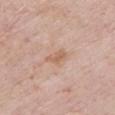Notes:
- workup · catalogued during a skin exam; not biopsied
- body site · the chest
- patient · female, aged approximately 70
- image · total-body-photography crop, ~15 mm field of view
- automated metrics · a footprint of about 3.5 mm², an outline eccentricity of about 0.85 (0 = round, 1 = elongated), and a symmetry-axis asymmetry near 0.3; a normalized border contrast of about 6; a color-variation rating of about 1.5/10 and peripheral color asymmetry of about 0.5
- size · ~3 mm (longest diameter)
- lighting · white-light